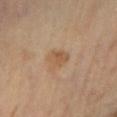Impression: This lesion was catalogued during total-body skin photography and was not selected for biopsy. Clinical summary: Located on the leg. A female patient, aged 68 to 72. Measured at roughly 2.5 mm in maximum diameter. This image is a 15 mm lesion crop taken from a total-body photograph.